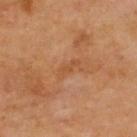– notes — no biopsy performed (imaged during a skin exam)
– tile lighting — cross-polarized
– diameter — ~3 mm (longest diameter)
– location — the upper back
– image source — total-body-photography crop, ~15 mm field of view
– patient — male, aged 68–72
– automated lesion analysis — a footprint of about 3 mm², an eccentricity of roughly 0.9, and two-axis asymmetry of about 0.4; a border-irregularity rating of about 4/10, a color-variation rating of about 0/10, and radial color variation of about 0